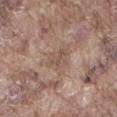<tbp_lesion>
<biopsy_status>not biopsied; imaged during a skin examination</biopsy_status>
<image>
  <source>total-body photography crop</source>
  <field_of_view_mm>15</field_of_view_mm>
</image>
<lighting>white-light</lighting>
<automated_metrics>
  <area_mm2_approx>4.5</area_mm2_approx>
  <eccentricity>0.55</eccentricity>
  <shape_asymmetry>0.35</shape_asymmetry>
  <border_irregularity_0_10>4.0</border_irregularity_0_10>
  <color_variation_0_10>2.0</color_variation_0_10>
  <lesion_detection_confidence_0_100>80</lesion_detection_confidence_0_100>
</automated_metrics>
<lesion_size>
  <long_diameter_mm_approx>2.5</long_diameter_mm_approx>
</lesion_size>
<patient>
  <sex>male</sex>
  <age_approx>75</age_approx>
</patient>
<site>right thigh</site>
</tbp_lesion>